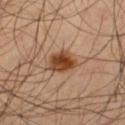Impression: Imaged during a routine full-body skin examination; the lesion was not biopsied and no histopathology is available. Image and clinical context: The lesion is on the leg. Automated image analysis of the tile measured a footprint of about 8 mm², an eccentricity of roughly 0.5, and a symmetry-axis asymmetry near 0.15. The software also gave a lesion color around L≈40 a*≈20 b*≈31 in CIELAB, a lesion–skin lightness drop of about 13, and a lesion-to-skin contrast of about 11 (normalized; higher = more distinct). The analysis additionally found radial color variation of about 1.5. The software also gave lesion-presence confidence of about 100/100. A region of skin cropped from a whole-body photographic capture, roughly 15 mm wide. Measured at roughly 3.5 mm in maximum diameter. A male subject, aged 53 to 57.